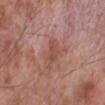{"biopsy_status": "not biopsied; imaged during a skin examination", "lesion_size": {"long_diameter_mm_approx": 2.5}, "image": {"source": "total-body photography crop", "field_of_view_mm": 15}, "lighting": "white-light", "site": "leg", "patient": {"sex": "male", "age_approx": 55}}